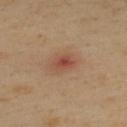workup: no biopsy performed (imaged during a skin exam)
lesion size: ≈3 mm
acquisition: ~15 mm tile from a whole-body skin photo
subject: male, aged 48–52
body site: the upper back